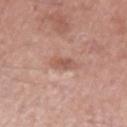| key | value |
|---|---|
| notes | catalogued during a skin exam; not biopsied |
| site | the left upper arm |
| imaging modality | ~15 mm crop, total-body skin-cancer survey |
| lesion size | ≈3 mm |
| patient | male, aged approximately 65 |